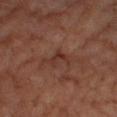Findings:
* workup · no biopsy performed (imaged during a skin exam)
* image-analysis metrics · a border-irregularity rating of about 6/10 and a peripheral color-asymmetry measure near 0; a classifier nevus-likeness of about 0/100 and lesion-presence confidence of about 70/100
* image source · total-body-photography crop, ~15 mm field of view
* size · ~3 mm (longest diameter)
* body site · the right lower leg
* lighting · cross-polarized
* patient · male, aged 58 to 62